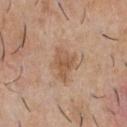  biopsy_status: not biopsied; imaged during a skin examination
  site: chest
  patient:
    sex: male
    age_approx: 30
  image:
    source: total-body photography crop
    field_of_view_mm: 15